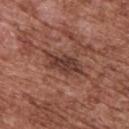Captured during whole-body skin photography for melanoma surveillance; the lesion was not biopsied.
The tile uses white-light illumination.
Approximately 5 mm at its widest.
A lesion tile, about 15 mm wide, cut from a 3D total-body photograph.
Automated tile analysis of the lesion measured a lesion area of about 8.5 mm². The analysis additionally found a mean CIELAB color near L≈38 a*≈22 b*≈24 and about 10 CIELAB-L* units darker than the surrounding skin. The software also gave a detector confidence of about 95 out of 100 that the crop contains a lesion.
On the upper back.
A male patient, in their mid-70s.